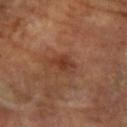The lesion was tiled from a total-body skin photograph and was not biopsied.
Cropped from a total-body skin-imaging series; the visible field is about 15 mm.
The subject is a female approximately 60 years of age.
The tile uses cross-polarized illumination.
Located on the left arm.
Automated image analysis of the tile measured a mean CIELAB color near L≈39 a*≈24 b*≈31, about 8 CIELAB-L* units darker than the surrounding skin, and a normalized lesion–skin contrast near 7.
About 3.5 mm across.